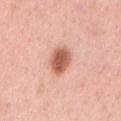Q: Was a biopsy performed?
A: catalogued during a skin exam; not biopsied
Q: Who is the patient?
A: male, in their mid-50s
Q: What is the imaging modality?
A: 15 mm crop, total-body photography
Q: Lesion size?
A: about 4 mm
Q: Automated lesion metrics?
A: a mean CIELAB color near L≈60 a*≈26 b*≈31, a lesion–skin lightness drop of about 16, and a lesion-to-skin contrast of about 10 (normalized; higher = more distinct)
Q: What is the anatomic site?
A: the mid back
Q: What lighting was used for the tile?
A: white-light illumination